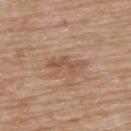Clinical impression:
The lesion was photographed on a routine skin check and not biopsied; there is no pathology result.
Background:
Measured at roughly 4.5 mm in maximum diameter. From the upper back. An algorithmic analysis of the crop reported a lesion area of about 6 mm² and a symmetry-axis asymmetry near 0.5. The software also gave a border-irregularity index near 6.5/10, internal color variation of about 1.5 on a 0–10 scale, and a peripheral color-asymmetry measure near 0.5. A 15 mm close-up extracted from a 3D total-body photography capture. The patient is a male about 85 years old or older. This is a white-light tile.